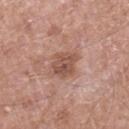No biopsy was performed on this lesion — it was imaged during a full skin examination and was not determined to be concerning.